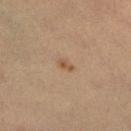Q: Was a biopsy performed?
A: catalogued during a skin exam; not biopsied
Q: How large is the lesion?
A: about 2 mm
Q: What is the imaging modality?
A: ~15 mm crop, total-body skin-cancer survey
Q: Patient demographics?
A: female, aged 28 to 32
Q: Automated lesion metrics?
A: an average lesion color of about L≈50 a*≈17 b*≈31 (CIELAB), roughly 8 lightness units darker than nearby skin, and a normalized border contrast of about 6.5; a border-irregularity rating of about 2.5/10, a within-lesion color-variation index near 0/10, and peripheral color asymmetry of about 0
Q: Where on the body is the lesion?
A: the right lower leg